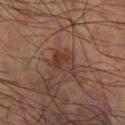Clinical impression: Imaged during a routine full-body skin examination; the lesion was not biopsied and no histopathology is available. Context: A 15 mm close-up tile from a total-body photography series done for melanoma screening. The tile uses cross-polarized illumination. A male subject aged 68–72. An algorithmic analysis of the crop reported an area of roughly 6.5 mm² and a symmetry-axis asymmetry near 0.3. The software also gave a mean CIELAB color near L≈29 a*≈17 b*≈21. It also reported a border-irregularity rating of about 3/10 and a peripheral color-asymmetry measure near 1. The analysis additionally found a classifier nevus-likeness of about 5/100 and a lesion-detection confidence of about 100/100. From the right lower leg. Approximately 3.5 mm at its widest.